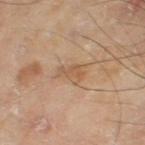– biopsy status — catalogued during a skin exam; not biopsied
– lighting — cross-polarized illumination
– patient — male, aged around 65
– body site — the right thigh
– lesion size — ≈3 mm
– image source — total-body-photography crop, ~15 mm field of view